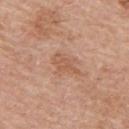Clinical impression: Imaged during a routine full-body skin examination; the lesion was not biopsied and no histopathology is available. Image and clinical context: Measured at roughly 3.5 mm in maximum diameter. Located on the upper back. The lesion-visualizer software estimated a lesion area of about 4.5 mm², an eccentricity of roughly 0.9, and a shape-asymmetry score of about 0.45 (0 = symmetric). The software also gave a border-irregularity index near 5/10 and radial color variation of about 0.5. The software also gave a nevus-likeness score of about 0/100 and a detector confidence of about 100 out of 100 that the crop contains a lesion. The subject is a male aged 58–62. Captured under white-light illumination. A 15 mm close-up tile from a total-body photography series done for melanoma screening.